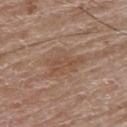workup = total-body-photography surveillance lesion; no biopsy
tile lighting = white-light illumination
imaging modality = ~15 mm tile from a whole-body skin photo
subject = male, aged around 85 or older
site = the arm
size = ≈5 mm